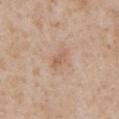Findings:
– notes: imaged on a skin check; not biopsied
– image: ~15 mm tile from a whole-body skin photo
– anatomic site: the chest
– patient: male, in their mid-60s